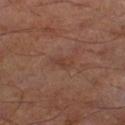Imaged during a routine full-body skin examination; the lesion was not biopsied and no histopathology is available.
From the right lower leg.
A region of skin cropped from a whole-body photographic capture, roughly 15 mm wide.
Automated tile analysis of the lesion measured an automated nevus-likeness rating near 0 out of 100 and a lesion-detection confidence of about 100/100.
A male patient, approximately 65 years of age.